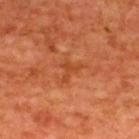Case summary:
- biopsy status · no biopsy performed (imaged during a skin exam)
- patient · male, roughly 65 years of age
- image-analysis metrics · a mean CIELAB color near L≈46 a*≈31 b*≈41, roughly 7 lightness units darker than nearby skin, and a lesion-to-skin contrast of about 5.5 (normalized; higher = more distinct); a border-irregularity index near 7/10, a color-variation rating of about 0/10, and peripheral color asymmetry of about 0; a classifier nevus-likeness of about 0/100 and a detector confidence of about 100 out of 100 that the crop contains a lesion
- site · the upper back
- lesion size · about 3 mm
- imaging modality · total-body-photography crop, ~15 mm field of view